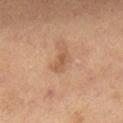Findings:
* notes · total-body-photography surveillance lesion; no biopsy
* illumination · cross-polarized
* TBP lesion metrics · about 7 CIELAB-L* units darker than the surrounding skin and a normalized border contrast of about 6; a border-irregularity index near 2.5/10 and peripheral color asymmetry of about 0
* image source · 15 mm crop, total-body photography
* lesion size · ≈3 mm
* subject · male, aged 53 to 57
* location · the lower back